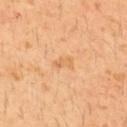Recorded during total-body skin imaging; not selected for excision or biopsy.
On the upper back.
Measured at roughly 2.5 mm in maximum diameter.
A 15 mm close-up extracted from a 3D total-body photography capture.
The subject is a male roughly 30 years of age.